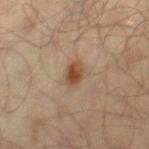This lesion was catalogued during total-body skin photography and was not selected for biopsy.
A male subject approximately 65 years of age.
From the right thigh.
Captured under cross-polarized illumination.
A lesion tile, about 15 mm wide, cut from a 3D total-body photograph.